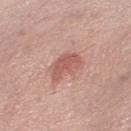| key | value |
|---|---|
| biopsy status | total-body-photography surveillance lesion; no biopsy |
| location | the left thigh |
| imaging modality | 15 mm crop, total-body photography |
| patient | female, aged approximately 40 |
| diameter | ~4 mm (longest diameter) |
| illumination | white-light illumination |
| image-analysis metrics | a lesion area of about 7 mm², an outline eccentricity of about 0.85 (0 = round, 1 = elongated), and two-axis asymmetry of about 0.35; a lesion color around L≈57 a*≈25 b*≈26 in CIELAB, about 10 CIELAB-L* units darker than the surrounding skin, and a normalized border contrast of about 6.5; a classifier nevus-likeness of about 40/100 and lesion-presence confidence of about 100/100 |